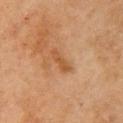Recorded during total-body skin imaging; not selected for excision or biopsy.
A female subject, aged approximately 70.
The tile uses cross-polarized illumination.
On the right upper arm.
Automated image analysis of the tile measured a footprint of about 4.5 mm² and an outline eccentricity of about 0.9 (0 = round, 1 = elongated). It also reported a border-irregularity rating of about 4/10, a color-variation rating of about 1.5/10, and a peripheral color-asymmetry measure near 0.5.
A 15 mm crop from a total-body photograph taken for skin-cancer surveillance.
Approximately 3.5 mm at its widest.